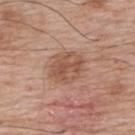Impression: The lesion was tiled from a total-body skin photograph and was not biopsied. Image and clinical context: The lesion is on the upper back. A lesion tile, about 15 mm wide, cut from a 3D total-body photograph. The subject is a male aged approximately 70.